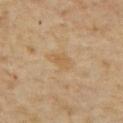Notes:
* workup — imaged on a skin check; not biopsied
* subject — male, aged 63–67
* image source — ~15 mm tile from a whole-body skin photo